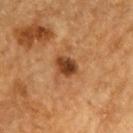The lesion was photographed on a routine skin check and not biopsied; there is no pathology result.
Measured at roughly 2.5 mm in maximum diameter.
The subject is a male in their mid- to late 80s or older.
The tile uses cross-polarized illumination.
The lesion is located on the head or neck.
A region of skin cropped from a whole-body photographic capture, roughly 15 mm wide.